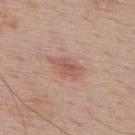Q: Was this lesion biopsied?
A: total-body-photography surveillance lesion; no biopsy
Q: Where on the body is the lesion?
A: the back
Q: Who is the patient?
A: male, in their 70s
Q: Automated lesion metrics?
A: roughly 9 lightness units darker than nearby skin; a border-irregularity index near 3.5/10, a color-variation rating of about 3/10, and peripheral color asymmetry of about 0.5
Q: What kind of image is this?
A: total-body-photography crop, ~15 mm field of view